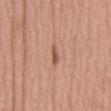<record>
<biopsy_status>not biopsied; imaged during a skin examination</biopsy_status>
<patient>
  <sex>male</sex>
  <age_approx>65</age_approx>
</patient>
<image>
  <source>total-body photography crop</source>
  <field_of_view_mm>15</field_of_view_mm>
</image>
<site>abdomen</site>
<lighting>white-light</lighting>
<lesion_size>
  <long_diameter_mm_approx>2.5</long_diameter_mm_approx>
</lesion_size>
</record>